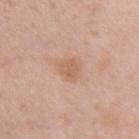Impression:
The lesion was photographed on a routine skin check and not biopsied; there is no pathology result.
Acquisition and patient details:
A female subject, about 40 years old. About 2.5 mm across. This image is a 15 mm lesion crop taken from a total-body photograph. The tile uses white-light illumination. The lesion is on the chest.